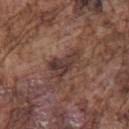<lesion>
<biopsy_status>not biopsied; imaged during a skin examination</biopsy_status>
<patient>
  <sex>male</sex>
  <age_approx>75</age_approx>
</patient>
<image>
  <source>total-body photography crop</source>
  <field_of_view_mm>15</field_of_view_mm>
</image>
<site>left upper arm</site>
</lesion>